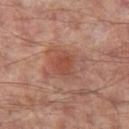Recorded during total-body skin imaging; not selected for excision or biopsy.
Approximately 3 mm at its widest.
This is a cross-polarized tile.
From the left thigh.
A roughly 15 mm field-of-view crop from a total-body skin photograph.
A male subject, aged 63 to 67.